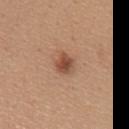Assessment: The lesion was tiled from a total-body skin photograph and was not biopsied. Background: On the back. The tile uses white-light illumination. A female subject, about 35 years old. A 15 mm crop from a total-body photograph taken for skin-cancer surveillance. Measured at roughly 2.5 mm in maximum diameter. Automated tile analysis of the lesion measured an outline eccentricity of about 0.45 (0 = round, 1 = elongated) and two-axis asymmetry of about 0.2. The software also gave internal color variation of about 4.5 on a 0–10 scale and peripheral color asymmetry of about 1.5. The analysis additionally found a nevus-likeness score of about 90/100 and lesion-presence confidence of about 100/100.